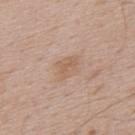| key | value |
|---|---|
| subject | male, roughly 70 years of age |
| TBP lesion metrics | an area of roughly 6.5 mm², an outline eccentricity of about 0.7 (0 = round, 1 = elongated), and two-axis asymmetry of about 0.25; a lesion color around L≈60 a*≈17 b*≈29 in CIELAB |
| lighting | white-light illumination |
| lesion diameter | ~3.5 mm (longest diameter) |
| imaging modality | ~15 mm crop, total-body skin-cancer survey |
| body site | the back |